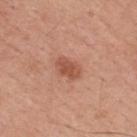The lesion was tiled from a total-body skin photograph and was not biopsied.
Longest diameter approximately 3 mm.
Imaged with white-light lighting.
On the upper back.
A lesion tile, about 15 mm wide, cut from a 3D total-body photograph.
The subject is a male approximately 55 years of age.